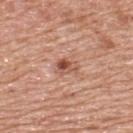Q: Is there a histopathology result?
A: no biopsy performed (imaged during a skin exam)